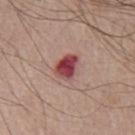notes = no biopsy performed (imaged during a skin exam)
diameter = ~3.5 mm (longest diameter)
patient = male, aged around 75
body site = the chest
illumination = white-light illumination
acquisition = ~15 mm tile from a whole-body skin photo